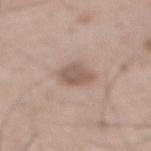This lesion was catalogued during total-body skin photography and was not selected for biopsy.